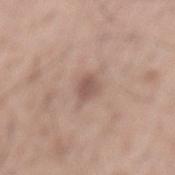The lesion was photographed on a routine skin check and not biopsied; there is no pathology result. The subject is a male in their 60s. From the mid back. Cropped from a whole-body photographic skin survey; the tile spans about 15 mm. Measured at roughly 2.5 mm in maximum diameter. Captured under white-light illumination.